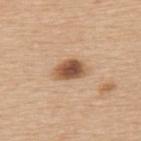Recorded during total-body skin imaging; not selected for excision or biopsy. From the upper back. A 15 mm close-up extracted from a 3D total-body photography capture. About 4 mm across. The patient is a female about 65 years old. The tile uses white-light illumination.